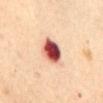Recorded during total-body skin imaging; not selected for excision or biopsy. The lesion is on the chest. Automated image analysis of the tile measured an area of roughly 9 mm², an outline eccentricity of about 0.55 (0 = round, 1 = elongated), and a shape-asymmetry score of about 0.25 (0 = symmetric). And it measured an average lesion color of about L≈55 a*≈31 b*≈28 (CIELAB) and about 25 CIELAB-L* units darker than the surrounding skin. The software also gave an automated nevus-likeness rating near 0 out of 100 and a detector confidence of about 100 out of 100 that the crop contains a lesion. Captured under cross-polarized illumination. A 15 mm crop from a total-body photograph taken for skin-cancer surveillance. A female patient, about 65 years old.